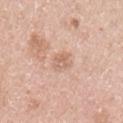The lesion-visualizer software estimated an area of roughly 3.5 mm² and two-axis asymmetry of about 0.35. And it measured a lesion–skin lightness drop of about 8 and a normalized border contrast of about 5.5. The software also gave a classifier nevus-likeness of about 0/100 and a detector confidence of about 100 out of 100 that the crop contains a lesion.
Approximately 2.5 mm at its widest.
A roughly 15 mm field-of-view crop from a total-body skin photograph.
Imaged with white-light lighting.
The lesion is on the left upper arm.
The subject is a female aged approximately 50.